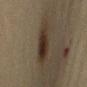Notes:
• workup · imaged on a skin check; not biopsied
• imaging modality · ~15 mm tile from a whole-body skin photo
• subject · female, in their mid-50s
• location · the right upper arm